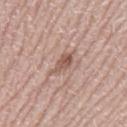- workup: imaged on a skin check; not biopsied
- body site: the right thigh
- patient: female, approximately 45 years of age
- imaging modality: ~15 mm crop, total-body skin-cancer survey
- diameter: ≈3.5 mm
- lighting: white-light illumination